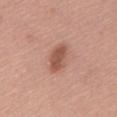Context:
The patient is a female roughly 65 years of age. Approximately 4 mm at its widest. The tile uses white-light illumination. The lesion-visualizer software estimated a footprint of about 7 mm², a shape eccentricity near 0.85, and two-axis asymmetry of about 0.25. The software also gave a lesion color around L≈54 a*≈24 b*≈28 in CIELAB, a lesion–skin lightness drop of about 11, and a normalized border contrast of about 7.5. It also reported a classifier nevus-likeness of about 80/100. From the chest. A lesion tile, about 15 mm wide, cut from a 3D total-body photograph.